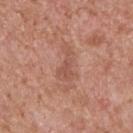{"biopsy_status": "not biopsied; imaged during a skin examination", "image": {"source": "total-body photography crop", "field_of_view_mm": 15}, "site": "upper back", "patient": {"sex": "male", "age_approx": 60}, "lighting": "white-light"}